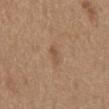biopsy_status: not biopsied; imaged during a skin examination
image:
  source: total-body photography crop
  field_of_view_mm: 15
patient:
  sex: male
  age_approx: 65
lighting: white-light
site: mid back
automated_metrics:
  cielab_L: 52
  cielab_a: 17
  cielab_b: 31
  vs_skin_darker_L: 7.0
  vs_skin_contrast_norm: 5.0
  border_irregularity_0_10: 4.5
  color_variation_0_10: 0.0
  peripheral_color_asymmetry: 0.0
  nevus_likeness_0_100: 0